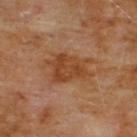biopsy_status: not biopsied; imaged during a skin examination
site: chest
automated_metrics:
  cielab_L: 42
  cielab_a: 22
  cielab_b: 35
  vs_skin_contrast_norm: 7.5
  border_irregularity_0_10: 4.0
  color_variation_0_10: 3.5
  peripheral_color_asymmetry: 1.0
patient:
  sex: male
  age_approx: 60
lighting: cross-polarized
lesion_size:
  long_diameter_mm_approx: 5.0
image:
  source: total-body photography crop
  field_of_view_mm: 15